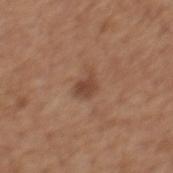Notes:
- biopsy status · catalogued during a skin exam; not biopsied
- location · the mid back
- lesion size · ~2.5 mm (longest diameter)
- patient · male, about 65 years old
- lighting · white-light
- image · 15 mm crop, total-body photography
- automated lesion analysis · a mean CIELAB color near L≈44 a*≈20 b*≈29, about 9 CIELAB-L* units darker than the surrounding skin, and a lesion-to-skin contrast of about 7 (normalized; higher = more distinct); a classifier nevus-likeness of about 40/100 and a detector confidence of about 100 out of 100 that the crop contains a lesion